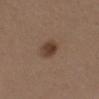| feature | finding |
|---|---|
| lesion diameter | ≈3 mm |
| image | 15 mm crop, total-body photography |
| illumination | white-light illumination |
| subject | female, aged 38 to 42 |
| anatomic site | the chest |